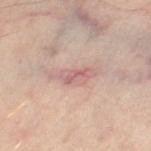The lesion was photographed on a routine skin check and not biopsied; there is no pathology result. The lesion is located on the right leg. The tile uses cross-polarized illumination. A female patient, approximately 40 years of age. A 15 mm close-up tile from a total-body photography series done for melanoma screening.